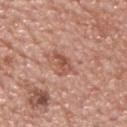Recorded during total-body skin imaging; not selected for excision or biopsy. A 15 mm crop from a total-body photograph taken for skin-cancer surveillance. Located on the upper back. Automated tile analysis of the lesion measured a footprint of about 4.5 mm² and an outline eccentricity of about 0.9 (0 = round, 1 = elongated). It also reported a lesion color around L≈53 a*≈25 b*≈29 in CIELAB and a lesion–skin lightness drop of about 10. The analysis additionally found border irregularity of about 4 on a 0–10 scale, a within-lesion color-variation index near 2.5/10, and a peripheral color-asymmetry measure near 0.5. And it measured a nevus-likeness score of about 0/100 and a detector confidence of about 100 out of 100 that the crop contains a lesion. This is a white-light tile. The recorded lesion diameter is about 3.5 mm. The subject is a male aged 73–77.